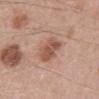Recorded during total-body skin imaging; not selected for excision or biopsy. The recorded lesion diameter is about 5 mm. Automated image analysis of the tile measured a lesion area of about 8.5 mm² and two-axis asymmetry of about 0.25. The software also gave border irregularity of about 3 on a 0–10 scale, a color-variation rating of about 3/10, and radial color variation of about 1. It also reported a nevus-likeness score of about 10/100 and a detector confidence of about 100 out of 100 that the crop contains a lesion. On the mid back. A 15 mm crop from a total-body photograph taken for skin-cancer surveillance. A male subject, in their 50s. Captured under white-light illumination.